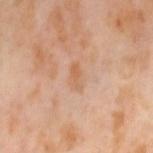Part of a total-body skin-imaging series; this lesion was reviewed on a skin check and was not flagged for biopsy.
Cropped from a total-body skin-imaging series; the visible field is about 15 mm.
A female subject about 55 years old.
The lesion-visualizer software estimated an area of roughly 4 mm², a shape eccentricity near 0.9, and a symmetry-axis asymmetry near 0.25. The software also gave a lesion color around L≈63 a*≈21 b*≈35 in CIELAB, a lesion–skin lightness drop of about 6, and a normalized lesion–skin contrast near 5.5. The software also gave a border-irregularity index near 2.5/10 and a peripheral color-asymmetry measure near 0.5.
Located on the leg.
This is a cross-polarized tile.
Measured at roughly 3 mm in maximum diameter.